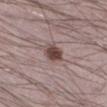| field | value |
|---|---|
| notes | catalogued during a skin exam; not biopsied |
| size | ≈2.5 mm |
| acquisition | 15 mm crop, total-body photography |
| subject | male, approximately 25 years of age |
| site | the right lower leg |
| automated lesion analysis | a lesion color around L≈44 a*≈17 b*≈19 in CIELAB, roughly 14 lightness units darker than nearby skin, and a normalized lesion–skin contrast near 10.5; border irregularity of about 1.5 on a 0–10 scale, internal color variation of about 3 on a 0–10 scale, and peripheral color asymmetry of about 1 |